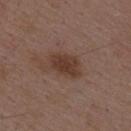Case summary:
• workup: catalogued during a skin exam; not biopsied
• subject: male, approximately 50 years of age
• illumination: white-light
• image: 15 mm crop, total-body photography
• location: the mid back
• automated lesion analysis: an area of roughly 7.5 mm² and a shape-asymmetry score of about 0.25 (0 = symmetric); a mean CIELAB color near L≈37 a*≈18 b*≈24 and about 9 CIELAB-L* units darker than the surrounding skin
• lesion size: about 4 mm